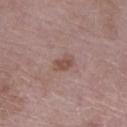Impression: The lesion was photographed on a routine skin check and not biopsied; there is no pathology result. Clinical summary: From the right lower leg. A region of skin cropped from a whole-body photographic capture, roughly 15 mm wide. The tile uses white-light illumination. A female subject in their 70s. Measured at roughly 2.5 mm in maximum diameter.